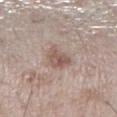Imaged during a routine full-body skin examination; the lesion was not biopsied and no histopathology is available. On the right lower leg. The lesion's longest dimension is about 3.5 mm. Captured under white-light illumination. A 15 mm crop from a total-body photograph taken for skin-cancer surveillance. An algorithmic analysis of the crop reported a footprint of about 6.5 mm² and two-axis asymmetry of about 0.25. And it measured roughly 10 lightness units darker than nearby skin. The analysis additionally found an automated nevus-likeness rating near 5 out of 100 and a detector confidence of about 100 out of 100 that the crop contains a lesion. The patient is a male aged approximately 60.